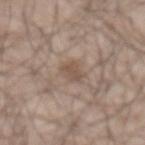Case summary:
* workup — catalogued during a skin exam; not biopsied
* lesion diameter — ≈2.5 mm
* location — the abdomen
* illumination — white-light
* subject — male, approximately 65 years of age
* image source — ~15 mm crop, total-body skin-cancer survey